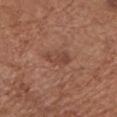{
  "site": "chest",
  "patient": {
    "sex": "female",
    "age_approx": 75
  },
  "image": {
    "source": "total-body photography crop",
    "field_of_view_mm": 15
  }
}